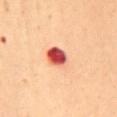{"lesion_size": {"long_diameter_mm_approx": 4.0}, "patient": {"sex": "female", "age_approx": 50}, "lighting": "cross-polarized", "image": {"source": "total-body photography crop", "field_of_view_mm": 15}, "site": "back", "automated_metrics": {"area_mm2_approx": 11.0, "eccentricity": 0.55, "shape_asymmetry": 0.15, "border_irregularity_0_10": 1.5, "color_variation_0_10": 10.0, "peripheral_color_asymmetry": 5.0, "nevus_likeness_0_100": 0}}